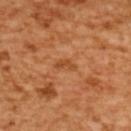{
  "lesion_size": {
    "long_diameter_mm_approx": 2.5
  },
  "image": {
    "source": "total-body photography crop",
    "field_of_view_mm": 15
  },
  "patient": {
    "sex": "female",
    "age_approx": 55
  },
  "site": "upper back"
}